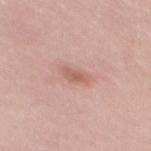The lesion was tiled from a total-body skin photograph and was not biopsied. From the mid back. A female patient roughly 25 years of age. A 15 mm close-up extracted from a 3D total-body photography capture. This is a white-light tile.